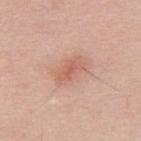This lesion was catalogued during total-body skin photography and was not selected for biopsy. The lesion is located on the upper back. A 15 mm crop from a total-body photograph taken for skin-cancer surveillance. A male subject, aged approximately 30. The lesion-visualizer software estimated a footprint of about 5 mm², an outline eccentricity of about 0.9 (0 = round, 1 = elongated), and two-axis asymmetry of about 0.25. The tile uses white-light illumination.